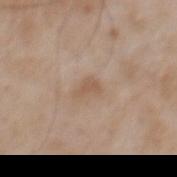Impression: Imaged during a routine full-body skin examination; the lesion was not biopsied and no histopathology is available. Clinical summary: A lesion tile, about 15 mm wide, cut from a 3D total-body photograph. A male subject about 50 years old. Longest diameter approximately 2.5 mm. Located on the mid back. An algorithmic analysis of the crop reported an automated nevus-likeness rating near 0 out of 100 and a lesion-detection confidence of about 100/100.